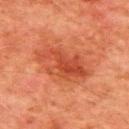<record>
  <biopsy_status>not biopsied; imaged during a skin examination</biopsy_status>
  <patient>
    <sex>male</sex>
    <age_approx>80</age_approx>
  </patient>
  <lighting>cross-polarized</lighting>
  <image>
    <source>total-body photography crop</source>
    <field_of_view_mm>15</field_of_view_mm>
  </image>
  <site>upper back</site>
</record>